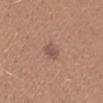The lesion was photographed on a routine skin check and not biopsied; there is no pathology result. A female patient, aged 18–22. A 15 mm crop from a total-body photograph taken for skin-cancer surveillance. From the right upper arm.